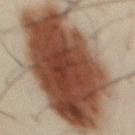notes = no biopsy performed (imaged during a skin exam) | acquisition = ~15 mm crop, total-body skin-cancer survey | site = the chest | subject = male, aged around 50 | lesion size = about 16.5 mm.